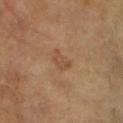No biopsy was performed on this lesion — it was imaged during a full skin examination and was not determined to be concerning. On the right upper arm. The subject is a female aged approximately 75. This image is a 15 mm lesion crop taken from a total-body photograph. Automated image analysis of the tile measured a footprint of about 4.5 mm², an outline eccentricity of about 0.8 (0 = round, 1 = elongated), and a shape-asymmetry score of about 0.3 (0 = symmetric). The software also gave border irregularity of about 3 on a 0–10 scale, internal color variation of about 2 on a 0–10 scale, and peripheral color asymmetry of about 0.5.